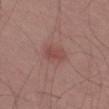| field | value |
|---|---|
| notes | catalogued during a skin exam; not biopsied |
| patient | male, approximately 55 years of age |
| acquisition | 15 mm crop, total-body photography |
| site | the left thigh |
| illumination | white-light illumination |
| lesion size | about 3 mm |